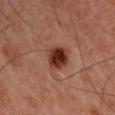follow-up: total-body-photography surveillance lesion; no biopsy | anatomic site: the right upper arm | acquisition: total-body-photography crop, ~15 mm field of view | subject: male, in their mid-40s | lesion diameter: ~4 mm (longest diameter) | tile lighting: cross-polarized.